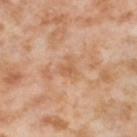{"patient": {"sex": "female", "age_approx": 55}, "image": {"source": "total-body photography crop", "field_of_view_mm": 15}, "lighting": "cross-polarized", "site": "left thigh"}